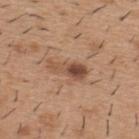Assessment: The lesion was photographed on a routine skin check and not biopsied; there is no pathology result. Image and clinical context: Cropped from a total-body skin-imaging series; the visible field is about 15 mm. An algorithmic analysis of the crop reported an average lesion color of about L≈49 a*≈20 b*≈30 (CIELAB) and a normalized lesion–skin contrast near 8.5. The analysis additionally found a border-irregularity rating of about 5/10 and a color-variation rating of about 7/10. The analysis additionally found a nevus-likeness score of about 65/100 and a detector confidence of about 100 out of 100 that the crop contains a lesion. On the back. A male subject, roughly 40 years of age. This is a white-light tile. The recorded lesion diameter is about 4.5 mm.